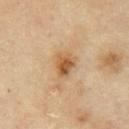| field | value |
|---|---|
| workup | imaged on a skin check; not biopsied |
| subject | male, aged 68 to 72 |
| lesion size | ~3 mm (longest diameter) |
| location | the abdomen |
| acquisition | ~15 mm crop, total-body skin-cancer survey |